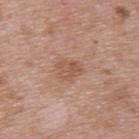<record>
<biopsy_status>not biopsied; imaged during a skin examination</biopsy_status>
<automated_metrics>
  <cielab_L>54</cielab_L>
  <cielab_a>20</cielab_a>
  <cielab_b>29</cielab_b>
  <vs_skin_darker_L>7.0</vs_skin_darker_L>
  <vs_skin_contrast_norm>5.5</vs_skin_contrast_norm>
  <border_irregularity_0_10>2.5</border_irregularity_0_10>
  <color_variation_0_10>3.5</color_variation_0_10>
  <peripheral_color_asymmetry>1.5</peripheral_color_asymmetry>
</automated_metrics>
<site>upper back</site>
<image>
  <source>total-body photography crop</source>
  <field_of_view_mm>15</field_of_view_mm>
</image>
<patient>
  <sex>male</sex>
  <age_approx>50</age_approx>
</patient>
<lighting>white-light</lighting>
</record>